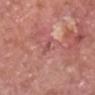Findings:
– patient — male, in their 60s
– imaging modality — 15 mm crop, total-body photography
– lesion diameter — ~1.5 mm (longest diameter)
– automated metrics — a footprint of about 1.5 mm², a shape eccentricity near 0.8, and a symmetry-axis asymmetry near 0.3; an average lesion color of about L≈51 a*≈27 b*≈24 (CIELAB), a lesion–skin lightness drop of about 8, and a lesion-to-skin contrast of about 5.5 (normalized; higher = more distinct); a nevus-likeness score of about 0/100 and a detector confidence of about 45 out of 100 that the crop contains a lesion
– body site — the head or neck
– biopsy diagnosis — a squamous cell carcinoma in situ (malignant)